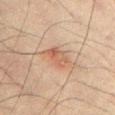follow-up: imaged on a skin check; not biopsied | lesion diameter: ≈3.5 mm | subject: male, approximately 70 years of age | imaging modality: 15 mm crop, total-body photography | body site: the front of the torso | automated lesion analysis: a border-irregularity index near 3/10, internal color variation of about 6 on a 0–10 scale, and a peripheral color-asymmetry measure near 2; an automated nevus-likeness rating near 40 out of 100 and a lesion-detection confidence of about 100/100 | tile lighting: cross-polarized.